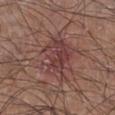Assessment: No biopsy was performed on this lesion — it was imaged during a full skin examination and was not determined to be concerning. Clinical summary: A region of skin cropped from a whole-body photographic capture, roughly 15 mm wide. The recorded lesion diameter is about 5 mm. On the right lower leg. The subject is a male in their mid- to late 40s.